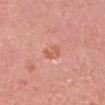follow-up: catalogued during a skin exam; not biopsied
automated lesion analysis: a footprint of about 3 mm², a shape eccentricity near 0.85, and a shape-asymmetry score of about 0.3 (0 = symmetric); a lesion color around L≈60 a*≈27 b*≈32 in CIELAB, roughly 8 lightness units darker than nearby skin, and a normalized lesion–skin contrast near 6
patient: female, aged approximately 40
lesion size: about 2.5 mm
tile lighting: white-light
location: the head or neck
image source: total-body-photography crop, ~15 mm field of view